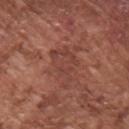The lesion was photographed on a routine skin check and not biopsied; there is no pathology result. Approximately 5.5 mm at its widest. The patient is a male roughly 75 years of age. On the arm. Automated image analysis of the tile measured a footprint of about 11 mm², an eccentricity of roughly 0.85, and two-axis asymmetry of about 0.4. It also reported a border-irregularity index near 5.5/10, internal color variation of about 3 on a 0–10 scale, and peripheral color asymmetry of about 1. The analysis additionally found a lesion-detection confidence of about 70/100. A roughly 15 mm field-of-view crop from a total-body skin photograph. Captured under white-light illumination.